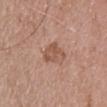biopsy_status: not biopsied; imaged during a skin examination
site: chest
automated_metrics:
  border_irregularity_0_10: 4.0
  color_variation_0_10: 2.5
  peripheral_color_asymmetry: 1.0
lighting: white-light
patient:
  sex: male
  age_approx: 70
image:
  source: total-body photography crop
  field_of_view_mm: 15